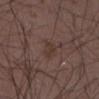  site: arm
  patient:
    sex: male
    age_approx: 50
  image:
    source: total-body photography crop
    field_of_view_mm: 15
  lighting: white-light
  lesion_size:
    long_diameter_mm_approx: 3.0
  automated_metrics:
    nevus_likeness_0_100: 0